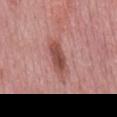| feature | finding |
|---|---|
| notes | total-body-photography surveillance lesion; no biopsy |
| image-analysis metrics | a mean CIELAB color near L≈50 a*≈26 b*≈25, a lesion–skin lightness drop of about 12, and a lesion-to-skin contrast of about 8.5 (normalized; higher = more distinct); a border-irregularity index near 2.5/10 and peripheral color asymmetry of about 1 |
| anatomic site | the mid back |
| patient | male, approximately 50 years of age |
| acquisition | total-body-photography crop, ~15 mm field of view |